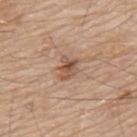Q: Was a biopsy performed?
A: imaged on a skin check; not biopsied
Q: What are the patient's age and sex?
A: male, aged around 80
Q: Lesion location?
A: the back
Q: What is the imaging modality?
A: total-body-photography crop, ~15 mm field of view
Q: What is the lesion's diameter?
A: ~3 mm (longest diameter)